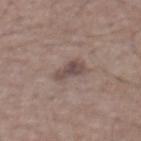Imaged during a routine full-body skin examination; the lesion was not biopsied and no histopathology is available. The lesion is located on the abdomen. A 15 mm close-up tile from a total-body photography series done for melanoma screening. The recorded lesion diameter is about 3 mm. A male subject about 55 years old.